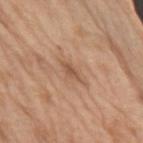This lesion was catalogued during total-body skin photography and was not selected for biopsy. A region of skin cropped from a whole-body photographic capture, roughly 15 mm wide. The total-body-photography lesion software estimated a border-irregularity index near 3/10 and peripheral color asymmetry of about 0.5. The tile uses white-light illumination. The lesion is on the right upper arm. A male subject approximately 70 years of age.